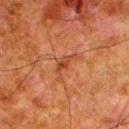The lesion was photographed on a routine skin check and not biopsied; there is no pathology result.
The recorded lesion diameter is about 3.5 mm.
The tile uses cross-polarized illumination.
The lesion-visualizer software estimated an area of roughly 4 mm², an outline eccentricity of about 0.85 (0 = round, 1 = elongated), and two-axis asymmetry of about 0.6.
The lesion is located on the left lower leg.
A male subject roughly 80 years of age.
A close-up tile cropped from a whole-body skin photograph, about 15 mm across.